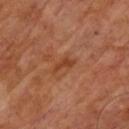Clinical impression:
Part of a total-body skin-imaging series; this lesion was reviewed on a skin check and was not flagged for biopsy.
Context:
The patient is a male roughly 65 years of age. Cropped from a total-body skin-imaging series; the visible field is about 15 mm. Measured at roughly 3 mm in maximum diameter. Captured under cross-polarized illumination. Automated tile analysis of the lesion measured a lesion color around L≈41 a*≈26 b*≈34 in CIELAB, roughly 8 lightness units darker than nearby skin, and a lesion-to-skin contrast of about 7 (normalized; higher = more distinct).